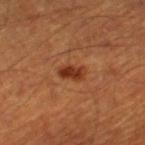biopsy status: total-body-photography surveillance lesion; no biopsy
acquisition: total-body-photography crop, ~15 mm field of view
subject: male, aged approximately 60
body site: the left thigh